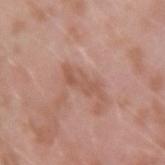workup: no biopsy performed (imaged during a skin exam) | imaging modality: ~15 mm tile from a whole-body skin photo | lesion size: ~4.5 mm (longest diameter) | body site: the arm | patient: male, aged 38–42 | tile lighting: white-light illumination | TBP lesion metrics: a lesion area of about 5.5 mm², an eccentricity of roughly 0.95, and a shape-asymmetry score of about 0.4 (0 = symmetric); a mean CIELAB color near L≈55 a*≈22 b*≈28, about 7 CIELAB-L* units darker than the surrounding skin, and a normalized border contrast of about 5.5; an automated nevus-likeness rating near 0 out of 100.